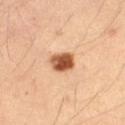{"biopsy_status": "not biopsied; imaged during a skin examination", "lesion_size": {"long_diameter_mm_approx": 3.0}, "image": {"source": "total-body photography crop", "field_of_view_mm": 15}, "patient": {"sex": "female", "age_approx": 35}, "automated_metrics": {"area_mm2_approx": 6.5, "eccentricity": 0.4, "shape_asymmetry": 0.2, "cielab_L": 56, "cielab_a": 25, "cielab_b": 38, "vs_skin_darker_L": 20.0, "vs_skin_contrast_norm": 12.0, "border_irregularity_0_10": 2.0, "peripheral_color_asymmetry": 2.0, "nevus_likeness_0_100": 100, "lesion_detection_confidence_0_100": 100}, "site": "left thigh"}